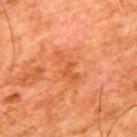Case summary:
– notes — imaged on a skin check; not biopsied
– image — ~15 mm crop, total-body skin-cancer survey
– patient — male, aged 78 to 82
– site — the upper back